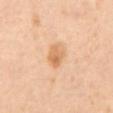{
  "biopsy_status": "not biopsied; imaged during a skin examination",
  "site": "abdomen",
  "lesion_size": {
    "long_diameter_mm_approx": 2.5
  },
  "lighting": "cross-polarized",
  "image": {
    "source": "total-body photography crop",
    "field_of_view_mm": 15
  },
  "automated_metrics": {
    "area_mm2_approx": 4.5,
    "eccentricity": 0.6,
    "shape_asymmetry": 0.25,
    "cielab_L": 69,
    "cielab_a": 22,
    "cielab_b": 41,
    "vs_skin_darker_L": 10.0,
    "vs_skin_contrast_norm": 7.0
  },
  "patient": {
    "sex": "female",
    "age_approx": 55
  }
}